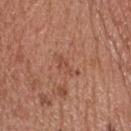This is a white-light tile.
The subject is a male aged 53 to 57.
From the head or neck.
The lesion's longest dimension is about 3.5 mm.
Automated image analysis of the tile measured an average lesion color of about L≈49 a*≈24 b*≈31 (CIELAB), roughly 6 lightness units darker than nearby skin, and a lesion-to-skin contrast of about 5 (normalized; higher = more distinct). The software also gave border irregularity of about 5.5 on a 0–10 scale, internal color variation of about 0.5 on a 0–10 scale, and a peripheral color-asymmetry measure near 0. The analysis additionally found a lesion-detection confidence of about 100/100.
A region of skin cropped from a whole-body photographic capture, roughly 15 mm wide.